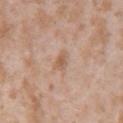workup: imaged on a skin check; not biopsied | automated metrics: a lesion area of about 3 mm² and a shape eccentricity near 0.8; a lesion color around L≈58 a*≈19 b*≈31 in CIELAB, about 8 CIELAB-L* units darker than the surrounding skin, and a lesion-to-skin contrast of about 6.5 (normalized; higher = more distinct); border irregularity of about 3 on a 0–10 scale, a color-variation rating of about 1.5/10, and a peripheral color-asymmetry measure near 0.5; an automated nevus-likeness rating near 0 out of 100 | subject: female, in their mid-20s | anatomic site: the left upper arm | imaging modality: total-body-photography crop, ~15 mm field of view | tile lighting: white-light | lesion diameter: ~2.5 mm (longest diameter).